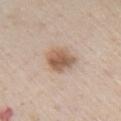Q: Is there a histopathology result?
A: total-body-photography surveillance lesion; no biopsy
Q: How was this image acquired?
A: total-body-photography crop, ~15 mm field of view
Q: What are the patient's age and sex?
A: female, aged 43–47
Q: Lesion location?
A: the chest
Q: Automated lesion metrics?
A: an outline eccentricity of about 0.6 (0 = round, 1 = elongated) and two-axis asymmetry of about 0.15; a mean CIELAB color near L≈59 a*≈17 b*≈29, roughly 12 lightness units darker than nearby skin, and a normalized border contrast of about 8.5; a border-irregularity rating of about 1.5/10, a color-variation rating of about 5/10, and peripheral color asymmetry of about 1.5
Q: How was the tile lit?
A: white-light